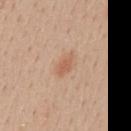Clinical impression:
This lesion was catalogued during total-body skin photography and was not selected for biopsy.
Background:
The lesion is located on the mid back. Approximately 2.5 mm at its widest. Captured under white-light illumination. A male subject, aged approximately 30. An algorithmic analysis of the crop reported a lesion area of about 3 mm² and an eccentricity of roughly 0.85. A roughly 15 mm field-of-view crop from a total-body skin photograph.